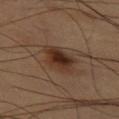Clinical summary: An algorithmic analysis of the crop reported an eccentricity of roughly 0.65 and a shape-asymmetry score of about 0.25 (0 = symmetric). It also reported a lesion color around L≈31 a*≈17 b*≈26 in CIELAB, a lesion–skin lightness drop of about 10, and a lesion-to-skin contrast of about 10 (normalized; higher = more distinct). And it measured a border-irregularity rating of about 2.5/10, a within-lesion color-variation index near 5.5/10, and radial color variation of about 1.5. The analysis additionally found a nevus-likeness score of about 90/100 and lesion-presence confidence of about 100/100. A region of skin cropped from a whole-body photographic capture, roughly 15 mm wide. Captured under cross-polarized illumination. A male subject, roughly 60 years of age. On the left thigh.